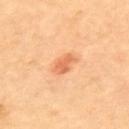Case summary:
* workup · imaged on a skin check; not biopsied
* image-analysis metrics · a lesion area of about 4 mm², an outline eccentricity of about 0.8 (0 = round, 1 = elongated), and a symmetry-axis asymmetry near 0.3; a nevus-likeness score of about 90/100 and a lesion-detection confidence of about 100/100
* size · about 2.5 mm
* image source · total-body-photography crop, ~15 mm field of view
* location · the upper back
* tile lighting · cross-polarized